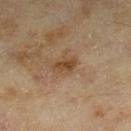workup = catalogued during a skin exam; not biopsied
lesion size = ≈3 mm
illumination = cross-polarized
automated metrics = a border-irregularity rating of about 3/10
subject = female, in their 60s
image source = ~15 mm tile from a whole-body skin photo
body site = the right lower leg